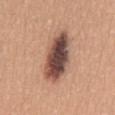Assessment:
Imaged during a routine full-body skin examination; the lesion was not biopsied and no histopathology is available.
Clinical summary:
About 7 mm across. This is a white-light tile. A region of skin cropped from a whole-body photographic capture, roughly 15 mm wide. The patient is a female approximately 30 years of age. On the mid back. The lesion-visualizer software estimated a footprint of about 17 mm². It also reported an average lesion color of about L≈47 a*≈20 b*≈24 (CIELAB), about 20 CIELAB-L* units darker than the surrounding skin, and a lesion-to-skin contrast of about 14 (normalized; higher = more distinct). The analysis additionally found a within-lesion color-variation index near 8.5/10 and radial color variation of about 2.5.